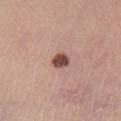The lesion-visualizer software estimated an area of roughly 4 mm², an outline eccentricity of about 0.7 (0 = round, 1 = elongated), and a shape-asymmetry score of about 0.25 (0 = symmetric). The software also gave a lesion–skin lightness drop of about 17 and a normalized border contrast of about 11.5. And it measured a classifier nevus-likeness of about 95/100 and lesion-presence confidence of about 100/100. Cropped from a whole-body photographic skin survey; the tile spans about 15 mm. Captured under white-light illumination. A female patient, approximately 60 years of age. The lesion is on the right lower leg.